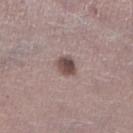Assessment: The lesion was tiled from a total-body skin photograph and was not biopsied. Clinical summary: The tile uses white-light illumination. From the right lower leg. Cropped from a whole-body photographic skin survey; the tile spans about 15 mm. The recorded lesion diameter is about 2.5 mm. A male patient, aged approximately 45.